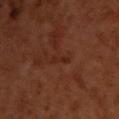<tbp_lesion>
  <automated_metrics>
    <eccentricity>0.95</eccentricity>
    <cielab_L>25</cielab_L>
    <cielab_a>22</cielab_a>
    <cielab_b>27</cielab_b>
    <vs_skin_darker_L>5.0</vs_skin_darker_L>
    <vs_skin_contrast_norm>5.5</vs_skin_contrast_norm>
  </automated_metrics>
  <patient>
    <sex>male</sex>
    <age_approx>50</age_approx>
  </patient>
  <lighting>cross-polarized</lighting>
  <image>
    <source>total-body photography crop</source>
    <field_of_view_mm>15</field_of_view_mm>
  </image>
  <site>upper back</site>
  <lesion_size>
    <long_diameter_mm_approx>3.0</long_diameter_mm_approx>
  </lesion_size>
</tbp_lesion>